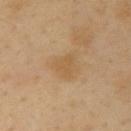biopsy status: no biopsy performed (imaged during a skin exam)
patient: male, about 40 years old
site: the right upper arm
diameter: about 2.5 mm
lighting: cross-polarized
acquisition: ~15 mm crop, total-body skin-cancer survey